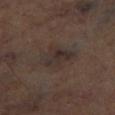Clinical impression: No biopsy was performed on this lesion — it was imaged during a full skin examination and was not determined to be concerning. Context: A lesion tile, about 15 mm wide, cut from a 3D total-body photograph. On the right lower leg. The total-body-photography lesion software estimated an eccentricity of roughly 0.5 and a symmetry-axis asymmetry near 0.25. The software also gave internal color variation of about 4 on a 0–10 scale and peripheral color asymmetry of about 1.5. The analysis additionally found lesion-presence confidence of about 90/100. This is a cross-polarized tile.